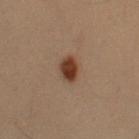<record>
<lesion_size>
  <long_diameter_mm_approx>3.0</long_diameter_mm_approx>
</lesion_size>
<automated_metrics>
  <nevus_likeness_0_100>100</nevus_likeness_0_100>
  <lesion_detection_confidence_0_100>100</lesion_detection_confidence_0_100>
</automated_metrics>
<image>
  <source>total-body photography crop</source>
  <field_of_view_mm>15</field_of_view_mm>
</image>
<patient>
  <sex>male</sex>
  <age_approx>55</age_approx>
</patient>
<lighting>cross-polarized</lighting>
<site>left upper arm</site>
</record>